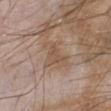{
  "biopsy_status": "not biopsied; imaged during a skin examination",
  "site": "left lower leg",
  "lesion_size": {
    "long_diameter_mm_approx": 3.0
  },
  "patient": {
    "sex": "male",
    "age_approx": 65
  },
  "image": {
    "source": "total-body photography crop",
    "field_of_view_mm": 15
  },
  "lighting": "white-light"
}